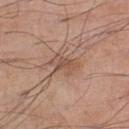A male patient, aged approximately 70. The lesion's longest dimension is about 3.5 mm. A close-up tile cropped from a whole-body skin photograph, about 15 mm across. The lesion is on the left lower leg. Imaged with white-light lighting.